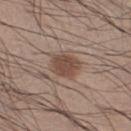| feature | finding |
|---|---|
| follow-up | imaged on a skin check; not biopsied |
| subject | male, aged 53–57 |
| lighting | white-light illumination |
| site | the right thigh |
| TBP lesion metrics | a footprint of about 8 mm² and a symmetry-axis asymmetry near 0.2; a border-irregularity index near 2/10, internal color variation of about 3 on a 0–10 scale, and a peripheral color-asymmetry measure near 1; a classifier nevus-likeness of about 100/100 and a lesion-detection confidence of about 100/100 |
| image | 15 mm crop, total-body photography |
| lesion diameter | ~3.5 mm (longest diameter) |